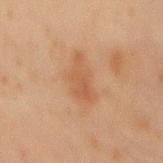This lesion was catalogued during total-body skin photography and was not selected for biopsy. A male subject aged approximately 45. On the mid back. The lesion's longest dimension is about 5.5 mm. Automated tile analysis of the lesion measured a footprint of about 11 mm² and an eccentricity of roughly 0.85. The software also gave a border-irregularity index near 3.5/10, a within-lesion color-variation index near 2/10, and radial color variation of about 0.5. A region of skin cropped from a whole-body photographic capture, roughly 15 mm wide. Imaged with cross-polarized lighting.